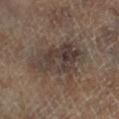<case>
  <biopsy_status>not biopsied; imaged during a skin examination</biopsy_status>
  <lighting>cross-polarized</lighting>
  <site>left lower leg</site>
  <patient>
    <sex>male</sex>
    <age_approx>65</age_approx>
  </patient>
  <image>
    <source>total-body photography crop</source>
    <field_of_view_mm>15</field_of_view_mm>
  </image>
  <automated_metrics>
    <area_mm2_approx>20.0</area_mm2_approx>
    <shape_asymmetry>0.3</shape_asymmetry>
    <cielab_L>35</cielab_L>
    <cielab_a>11</cielab_a>
    <cielab_b>18</cielab_b>
    <vs_skin_darker_L>8.0</vs_skin_darker_L>
    <vs_skin_contrast_norm>8.5</vs_skin_contrast_norm>
  </automated_metrics>
  <lesion_size>
    <long_diameter_mm_approx>7.0</long_diameter_mm_approx>
  </lesion_size>
</case>